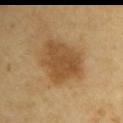Captured during whole-body skin photography for melanoma surveillance; the lesion was not biopsied. On the left upper arm. Cropped from a whole-body photographic skin survey; the tile spans about 15 mm. A male patient, aged 58–62. Approximately 6 mm at its widest. This is a cross-polarized tile.